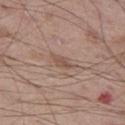{"biopsy_status": "not biopsied; imaged during a skin examination", "image": {"source": "total-body photography crop", "field_of_view_mm": 15}, "automated_metrics": {"border_irregularity_0_10": 3.0, "color_variation_0_10": 1.5}, "lighting": "white-light", "patient": {"sex": "male", "age_approx": 50}, "lesion_size": {"long_diameter_mm_approx": 3.5}, "site": "left thigh"}